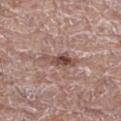Q: Lesion size?
A: ~4 mm (longest diameter)
Q: What kind of image is this?
A: ~15 mm crop, total-body skin-cancer survey
Q: What did automated image analysis measure?
A: a lesion color around L≈48 a*≈19 b*≈22 in CIELAB, about 12 CIELAB-L* units darker than the surrounding skin, and a normalized lesion–skin contrast near 8.5; a border-irregularity index near 4.5/10, a within-lesion color-variation index near 7.5/10, and a peripheral color-asymmetry measure near 3; a nevus-likeness score of about 90/100 and lesion-presence confidence of about 100/100
Q: Patient demographics?
A: male, aged approximately 70
Q: Lesion location?
A: the right thigh
Q: Illumination type?
A: white-light illumination